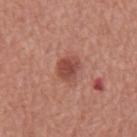Recorded during total-body skin imaging; not selected for excision or biopsy. A 15 mm close-up extracted from a 3D total-body photography capture. A male patient about 65 years old. The lesion is located on the mid back. Automated image analysis of the tile measured an area of roughly 6 mm² and two-axis asymmetry of about 0.25. The analysis additionally found a mean CIELAB color near L≈48 a*≈27 b*≈28, a lesion–skin lightness drop of about 11, and a normalized border contrast of about 8. The software also gave a detector confidence of about 100 out of 100 that the crop contains a lesion. The tile uses white-light illumination.